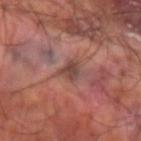Impression:
Imaged during a routine full-body skin examination; the lesion was not biopsied and no histopathology is available.
Context:
A lesion tile, about 15 mm wide, cut from a 3D total-body photograph. The lesion is on the left forearm. The subject is a male aged 58–62. Longest diameter approximately 2.5 mm. This is a cross-polarized tile.